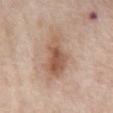Clinical impression: The lesion was tiled from a total-body skin photograph and was not biopsied. Background: Approximately 7 mm at its widest. A female patient aged around 60. The lesion-visualizer software estimated an eccentricity of roughly 0.9 and a shape-asymmetry score of about 0.3 (0 = symmetric). And it measured a within-lesion color-variation index near 6.5/10 and a peripheral color-asymmetry measure near 2. The analysis additionally found a nevus-likeness score of about 65/100 and a lesion-detection confidence of about 100/100. The lesion is on the abdomen. This image is a 15 mm lesion crop taken from a total-body photograph. This is a white-light tile.